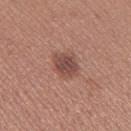The lesion was tiled from a total-body skin photograph and was not biopsied. Automated tile analysis of the lesion measured a lesion–skin lightness drop of about 11. The software also gave border irregularity of about 1.5 on a 0–10 scale and a peripheral color-asymmetry measure near 1. The software also gave a classifier nevus-likeness of about 55/100. A region of skin cropped from a whole-body photographic capture, roughly 15 mm wide. Imaged with white-light lighting. The patient is a female about 30 years old. The recorded lesion diameter is about 3.5 mm. Located on the leg.